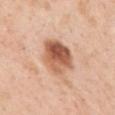biopsy status: no biopsy performed (imaged during a skin exam); patient: female, roughly 50 years of age; anatomic site: the mid back; acquisition: total-body-photography crop, ~15 mm field of view.